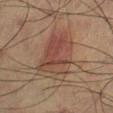Recorded during total-body skin imaging; not selected for excision or biopsy. The lesion's longest dimension is about 6.5 mm. A 15 mm close-up extracted from a 3D total-body photography capture. The patient is a male aged 58–62. The tile uses cross-polarized illumination. The total-body-photography lesion software estimated an automated nevus-likeness rating near 70 out of 100 and a detector confidence of about 100 out of 100 that the crop contains a lesion.